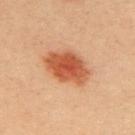Imaged during a routine full-body skin examination; the lesion was not biopsied and no histopathology is available. From the upper back. A female patient aged approximately 35. An algorithmic analysis of the crop reported a footprint of about 15 mm² and a symmetry-axis asymmetry near 0.15. It also reported a lesion color around L≈55 a*≈29 b*≈38 in CIELAB and a normalized lesion–skin contrast near 9.5. And it measured a border-irregularity rating of about 1.5/10, a color-variation rating of about 4.5/10, and peripheral color asymmetry of about 1. The recorded lesion diameter is about 5.5 mm. A roughly 15 mm field-of-view crop from a total-body skin photograph. Captured under cross-polarized illumination.